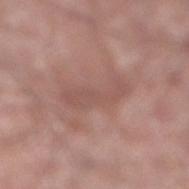follow-up: imaged on a skin check; not biopsied | patient: male, aged around 60 | anatomic site: the leg | diameter: ≈6.5 mm | image source: total-body-photography crop, ~15 mm field of view | lighting: white-light illumination.